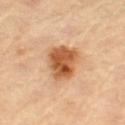This is a cross-polarized tile. A female subject about 70 years old. The lesion is located on the left thigh. A close-up tile cropped from a whole-body skin photograph, about 15 mm across. Measured at roughly 5.5 mm in maximum diameter.